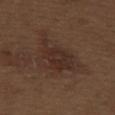Q: Was a biopsy performed?
A: no biopsy performed (imaged during a skin exam)
Q: What is the anatomic site?
A: the upper back
Q: What are the patient's age and sex?
A: male, about 70 years old
Q: How large is the lesion?
A: ~6 mm (longest diameter)
Q: What did automated image analysis measure?
A: an outline eccentricity of about 0.85 (0 = round, 1 = elongated) and a symmetry-axis asymmetry near 0.35; a mean CIELAB color near L≈29 a*≈16 b*≈22 and about 6 CIELAB-L* units darker than the surrounding skin; an automated nevus-likeness rating near 0 out of 100 and a lesion-detection confidence of about 100/100
Q: How was this image acquired?
A: ~15 mm tile from a whole-body skin photo